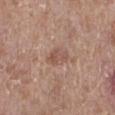| key | value |
|---|---|
| notes | catalogued during a skin exam; not biopsied |
| lighting | white-light |
| diameter | ~3 mm (longest diameter) |
| subject | male, approximately 80 years of age |
| acquisition | total-body-photography crop, ~15 mm field of view |
| body site | the left leg |